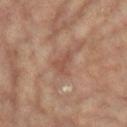Q: Was this lesion biopsied?
A: imaged on a skin check; not biopsied
Q: What is the imaging modality?
A: ~15 mm tile from a whole-body skin photo
Q: How was the tile lit?
A: cross-polarized illumination
Q: What is the anatomic site?
A: the left lower leg
Q: Automated lesion metrics?
A: an outline eccentricity of about 0.75 (0 = round, 1 = elongated) and a shape-asymmetry score of about 0.45 (0 = symmetric); a lesion color around L≈50 a*≈22 b*≈28 in CIELAB, a lesion–skin lightness drop of about 7, and a normalized border contrast of about 5.5; border irregularity of about 4.5 on a 0–10 scale, a within-lesion color-variation index near 1.5/10, and radial color variation of about 0.5; a nevus-likeness score of about 0/100 and a lesion-detection confidence of about 100/100
Q: What are the patient's age and sex?
A: female, aged 73–77
Q: Lesion size?
A: ~3 mm (longest diameter)